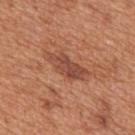<case>
<biopsy_status>not biopsied; imaged during a skin examination</biopsy_status>
<site>back</site>
<patient>
  <sex>male</sex>
  <age_approx>65</age_approx>
</patient>
<lighting>white-light</lighting>
<lesion_size>
  <long_diameter_mm_approx>5.0</long_diameter_mm_approx>
</lesion_size>
<image>
  <source>total-body photography crop</source>
  <field_of_view_mm>15</field_of_view_mm>
</image>
</case>